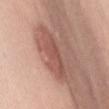Clinical impression: No biopsy was performed on this lesion — it was imaged during a full skin examination and was not determined to be concerning. Background: A 15 mm close-up extracted from a 3D total-body photography capture. From the chest. Automated image analysis of the tile measured a mean CIELAB color near L≈53 a*≈23 b*≈26, a lesion–skin lightness drop of about 10, and a normalized border contrast of about 7. A female patient approximately 60 years of age. Imaged with white-light lighting.